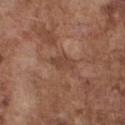Assessment:
Captured during whole-body skin photography for melanoma surveillance; the lesion was not biopsied.
Image and clinical context:
The lesion is located on the chest. Imaged with white-light lighting. The recorded lesion diameter is about 3 mm. A close-up tile cropped from a whole-body skin photograph, about 15 mm across. The subject is a male approximately 75 years of age. The total-body-photography lesion software estimated a lesion area of about 4 mm² and a symmetry-axis asymmetry near 0.3.